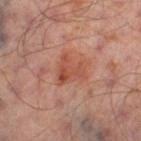workup: no biopsy performed (imaged during a skin exam).